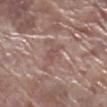{"lesion_size": {"long_diameter_mm_approx": 2.5}, "image": {"source": "total-body photography crop", "field_of_view_mm": 15}, "lighting": "white-light", "site": "right lower leg", "patient": {"sex": "male", "age_approx": 70}}